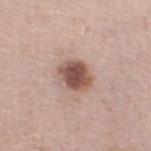| field | value |
|---|---|
| workup | imaged on a skin check; not biopsied |
| body site | the left thigh |
| tile lighting | white-light |
| patient | male, in their mid- to late 60s |
| lesion size | about 3.5 mm |
| acquisition | total-body-photography crop, ~15 mm field of view |